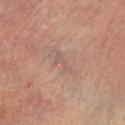  lesion_size:
    long_diameter_mm_approx: 3.0
  site: left lower leg
  image:
    source: total-body photography crop
    field_of_view_mm: 15
  lighting: cross-polarized
  patient:
    sex: male
    age_approx: 75